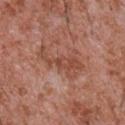| feature | finding |
|---|---|
| workup | no biopsy performed (imaged during a skin exam) |
| imaging modality | 15 mm crop, total-body photography |
| illumination | white-light illumination |
| patient | male, about 45 years old |
| body site | the chest |